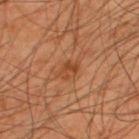Assessment:
This lesion was catalogued during total-body skin photography and was not selected for biopsy.
Acquisition and patient details:
A region of skin cropped from a whole-body photographic capture, roughly 15 mm wide. The tile uses cross-polarized illumination. An algorithmic analysis of the crop reported a footprint of about 4 mm², a shape eccentricity near 0.7, and two-axis asymmetry of about 0.2. The software also gave a mean CIELAB color near L≈43 a*≈25 b*≈36, about 8 CIELAB-L* units darker than the surrounding skin, and a lesion-to-skin contrast of about 6.5 (normalized; higher = more distinct). The analysis additionally found a color-variation rating of about 4/10 and a peripheral color-asymmetry measure near 1.5. The software also gave a classifier nevus-likeness of about 30/100 and lesion-presence confidence of about 100/100. The lesion is on the back. Approximately 2.5 mm at its widest. A male subject in their mid- to late 40s.